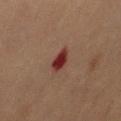* biopsy status — imaged on a skin check; not biopsied
* imaging modality — total-body-photography crop, ~15 mm field of view
* anatomic site — the mid back
* subject — female, roughly 80 years of age
* automated metrics — a footprint of about 4 mm², an eccentricity of roughly 0.65, and a symmetry-axis asymmetry near 0.2
* lighting — cross-polarized
* lesion diameter — ≈2.5 mm